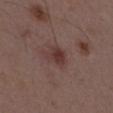Clinical impression: Imaged during a routine full-body skin examination; the lesion was not biopsied and no histopathology is available. Clinical summary: From the mid back. A 15 mm close-up tile from a total-body photography series done for melanoma screening. The total-body-photography lesion software estimated a lesion area of about 5.5 mm² and a shape-asymmetry score of about 0.3 (0 = symmetric). The analysis additionally found an average lesion color of about L≈35 a*≈20 b*≈20 (CIELAB), a lesion–skin lightness drop of about 8, and a normalized border contrast of about 7.5. It also reported a border-irregularity rating of about 2.5/10, a color-variation rating of about 3.5/10, and peripheral color asymmetry of about 1. Approximately 3 mm at its widest. The tile uses white-light illumination. A male patient aged approximately 50.